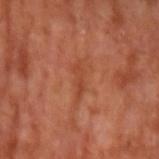<record>
<biopsy_status>not biopsied; imaged during a skin examination</biopsy_status>
<site>left upper arm</site>
<patient>
  <sex>male</sex>
  <age_approx>55</age_approx>
</patient>
<lighting>cross-polarized</lighting>
<lesion_size>
  <long_diameter_mm_approx>5.0</long_diameter_mm_approx>
</lesion_size>
<image>
  <source>total-body photography crop</source>
  <field_of_view_mm>15</field_of_view_mm>
</image>
<automated_metrics>
  <area_mm2_approx>6.0</area_mm2_approx>
  <eccentricity>0.95</eccentricity>
  <shape_asymmetry>0.45</shape_asymmetry>
  <nevus_likeness_0_100>0</nevus_likeness_0_100>
  <lesion_detection_confidence_0_100>65</lesion_detection_confidence_0_100>
</automated_metrics>
</record>